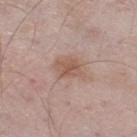Context: A male subject approximately 55 years of age. The lesion is located on the leg. A lesion tile, about 15 mm wide, cut from a 3D total-body photograph.